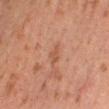– biopsy status: catalogued during a skin exam; not biopsied
– imaging modality: 15 mm crop, total-body photography
– TBP lesion metrics: roughly 6 lightness units darker than nearby skin and a lesion-to-skin contrast of about 5.5 (normalized; higher = more distinct); a border-irregularity rating of about 3/10 and radial color variation of about 0.5; a classifier nevus-likeness of about 0/100 and lesion-presence confidence of about 100/100
– diameter: about 2.5 mm
– lighting: cross-polarized
– site: the leg
– subject: female, aged 18 to 22